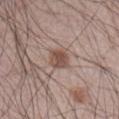<tbp_lesion>
  <automated_metrics>
    <area_mm2_approx>5.5</area_mm2_approx>
    <eccentricity>0.5</eccentricity>
    <border_irregularity_0_10>1.5</border_irregularity_0_10>
    <color_variation_0_10>3.0</color_variation_0_10>
    <peripheral_color_asymmetry>1.0</peripheral_color_asymmetry>
  </automated_metrics>
  <site>abdomen</site>
  <patient>
    <sex>male</sex>
    <age_approx>65</age_approx>
  </patient>
  <image>
    <source>total-body photography crop</source>
    <field_of_view_mm>15</field_of_view_mm>
  </image>
  <lesion_size>
    <long_diameter_mm_approx>2.5</long_diameter_mm_approx>
  </lesion_size>
</tbp_lesion>